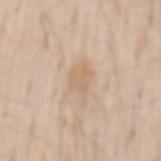Imaged during a routine full-body skin examination; the lesion was not biopsied and no histopathology is available.
Located on the mid back.
An algorithmic analysis of the crop reported an average lesion color of about L≈66 a*≈15 b*≈32 (CIELAB), a lesion–skin lightness drop of about 7, and a normalized lesion–skin contrast near 5. And it measured a border-irregularity rating of about 4.5/10, internal color variation of about 1.5 on a 0–10 scale, and radial color variation of about 0.5. The software also gave a classifier nevus-likeness of about 0/100 and a detector confidence of about 100 out of 100 that the crop contains a lesion.
Approximately 4 mm at its widest.
A male patient aged around 60.
Captured under white-light illumination.
A 15 mm close-up extracted from a 3D total-body photography capture.